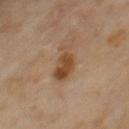The lesion was photographed on a routine skin check and not biopsied; there is no pathology result. The subject is a male aged 58 to 62. On the mid back. Approximately 4.5 mm at its widest. Captured under cross-polarized illumination. A roughly 15 mm field-of-view crop from a total-body skin photograph.